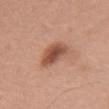{"biopsy_status": "not biopsied; imaged during a skin examination", "patient": {"sex": "female", "age_approx": 65}, "lesion_size": {"long_diameter_mm_approx": 3.5}, "image": {"source": "total-body photography crop", "field_of_view_mm": 15}, "lighting": "white-light", "site": "chest"}